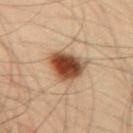biopsy status=total-body-photography surveillance lesion; no biopsy | acquisition=~15 mm crop, total-body skin-cancer survey | patient=male, aged approximately 35 | illumination=cross-polarized | diameter=about 4 mm | automated lesion analysis=a detector confidence of about 100 out of 100 that the crop contains a lesion | body site=the right upper arm.